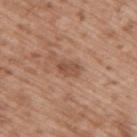Imaged during a routine full-body skin examination; the lesion was not biopsied and no histopathology is available. Captured under white-light illumination. A 15 mm crop from a total-body photograph taken for skin-cancer surveillance. A male patient, aged around 50. Located on the arm.